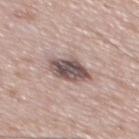The lesion was photographed on a routine skin check and not biopsied; there is no pathology result. The subject is a male in their mid- to late 60s. On the mid back. A 15 mm close-up tile from a total-body photography series done for melanoma screening.